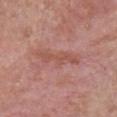Assessment:
Imaged during a routine full-body skin examination; the lesion was not biopsied and no histopathology is available.
Image and clinical context:
This is a white-light tile. A male subject, roughly 40 years of age. On the chest. A 15 mm close-up tile from a total-body photography series done for melanoma screening. The total-body-photography lesion software estimated an average lesion color of about L≈52 a*≈25 b*≈27 (CIELAB), about 7 CIELAB-L* units darker than the surrounding skin, and a normalized lesion–skin contrast near 6.